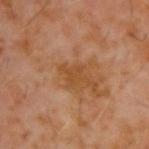follow-up: imaged on a skin check; not biopsied | acquisition: total-body-photography crop, ~15 mm field of view | patient: male, roughly 60 years of age | automated metrics: a lesion area of about 3.5 mm² and a shape-asymmetry score of about 0.4 (0 = symmetric); a border-irregularity index near 5/10, internal color variation of about 1 on a 0–10 scale, and a peripheral color-asymmetry measure near 0.5 | lesion size: about 3 mm | lighting: cross-polarized illumination | location: the upper back.